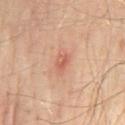biopsy_status: not biopsied; imaged during a skin examination
patient:
  age_approx: 55
image:
  source: total-body photography crop
  field_of_view_mm: 15
lighting: cross-polarized
lesion_size:
  long_diameter_mm_approx: 2.5
site: mid back